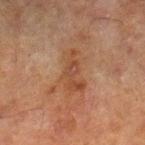The lesion was tiled from a total-body skin photograph and was not biopsied.
A male subject, aged 63–67.
This is a cross-polarized tile.
On the left lower leg.
The lesion-visualizer software estimated a border-irregularity index near 5.5/10, internal color variation of about 3 on a 0–10 scale, and radial color variation of about 1.
This image is a 15 mm lesion crop taken from a total-body photograph.